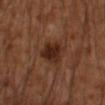tile lighting=cross-polarized
lesion size=≈4 mm
image source=~15 mm tile from a whole-body skin photo
anatomic site=the right forearm
patient=male, in their mid- to late 40s
TBP lesion metrics=a normalized border contrast of about 10